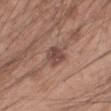biopsy status — no biopsy performed (imaged during a skin exam)
patient — male, in their 20s
imaging modality — ~15 mm crop, total-body skin-cancer survey
location — the left forearm